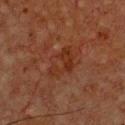No biopsy was performed on this lesion — it was imaged during a full skin examination and was not determined to be concerning. The lesion is located on the chest. The subject is a male about 65 years old. A lesion tile, about 15 mm wide, cut from a 3D total-body photograph.